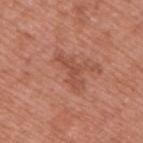No biopsy was performed on this lesion — it was imaged during a full skin examination and was not determined to be concerning.
On the upper back.
Imaged with white-light lighting.
The lesion's longest dimension is about 4.5 mm.
A male patient, approximately 70 years of age.
A 15 mm close-up extracted from a 3D total-body photography capture.